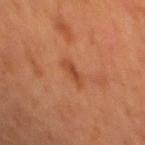Clinical impression:
Imaged during a routine full-body skin examination; the lesion was not biopsied and no histopathology is available.
Clinical summary:
This is a cross-polarized tile. A 15 mm close-up extracted from a 3D total-body photography capture. A male subject roughly 65 years of age. Measured at roughly 3 mm in maximum diameter. The lesion-visualizer software estimated an average lesion color of about L≈42 a*≈26 b*≈34 (CIELAB), roughly 7 lightness units darker than nearby skin, and a normalized border contrast of about 6.5. And it measured border irregularity of about 3.5 on a 0–10 scale, internal color variation of about 1 on a 0–10 scale, and a peripheral color-asymmetry measure near 0. The analysis additionally found an automated nevus-likeness rating near 25 out of 100. The lesion is located on the back.